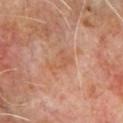follow-up = no biopsy performed (imaged during a skin exam).